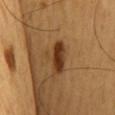| feature | finding |
|---|---|
| workup | total-body-photography surveillance lesion; no biopsy |
| subject | male, about 60 years old |
| lesion diameter | about 5 mm |
| automated metrics | a footprint of about 8.5 mm², a shape eccentricity near 0.9, and a shape-asymmetry score of about 0.3 (0 = symmetric); an average lesion color of about L≈37 a*≈20 b*≈34 (CIELAB), a lesion–skin lightness drop of about 13, and a lesion-to-skin contrast of about 10.5 (normalized; higher = more distinct) |
| site | the mid back |
| imaging modality | ~15 mm tile from a whole-body skin photo |